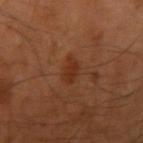Q: What did automated image analysis measure?
A: an average lesion color of about L≈31 a*≈24 b*≈31 (CIELAB), about 7 CIELAB-L* units darker than the surrounding skin, and a normalized border contrast of about 6.5; a border-irregularity rating of about 3/10, a within-lesion color-variation index near 2/10, and radial color variation of about 0.5; a nevus-likeness score of about 30/100 and a detector confidence of about 100 out of 100 that the crop contains a lesion
Q: What are the patient's age and sex?
A: male, aged 48–52
Q: What kind of image is this?
A: ~15 mm tile from a whole-body skin photo
Q: Illumination type?
A: cross-polarized illumination
Q: How large is the lesion?
A: ~2.5 mm (longest diameter)
Q: What is the anatomic site?
A: the left arm